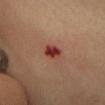Recorded during total-body skin imaging; not selected for excision or biopsy.
The total-body-photography lesion software estimated a footprint of about 4.5 mm², a shape eccentricity near 0.55, and two-axis asymmetry of about 0.25. The software also gave a border-irregularity rating of about 2/10, a color-variation rating of about 4/10, and a peripheral color-asymmetry measure near 1. The software also gave a classifier nevus-likeness of about 0/100 and a lesion-detection confidence of about 100/100.
Imaged with cross-polarized lighting.
Located on the head or neck.
A roughly 15 mm field-of-view crop from a total-body skin photograph.
A female subject aged around 40.